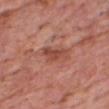<tbp_lesion>
  <biopsy_status>not biopsied; imaged during a skin examination</biopsy_status>
  <automated_metrics>
    <eccentricity>0.9</eccentricity>
    <vs_skin_darker_L>10.0</vs_skin_darker_L>
    <vs_skin_contrast_norm>7.0</vs_skin_contrast_norm>
  </automated_metrics>
  <patient>
    <sex>male</sex>
    <age_approx>60</age_approx>
  </patient>
  <lighting>white-light</lighting>
  <lesion_size>
    <long_diameter_mm_approx>4.0</long_diameter_mm_approx>
  </lesion_size>
  <site>chest</site>
  <image>
    <source>total-body photography crop</source>
    <field_of_view_mm>15</field_of_view_mm>
  </image>
</tbp_lesion>